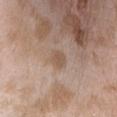The lesion is on the head or neck. A 15 mm crop from a total-body photograph taken for skin-cancer surveillance. The patient is a female aged 23 to 27.